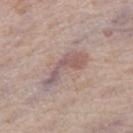The lesion was tiled from a total-body skin photograph and was not biopsied. Located on the right thigh. About 6 mm across. A roughly 15 mm field-of-view crop from a total-body skin photograph. Imaged with white-light lighting. A female patient, in their 60s.